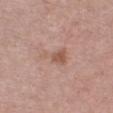- size · ~4 mm (longest diameter)
- TBP lesion metrics · a lesion area of about 6.5 mm², an outline eccentricity of about 0.85 (0 = round, 1 = elongated), and two-axis asymmetry of about 0.5; a border-irregularity index near 5/10 and radial color variation of about 1; a nevus-likeness score of about 5/100 and a detector confidence of about 100 out of 100 that the crop contains a lesion
- body site · the chest
- tile lighting · white-light
- image source · ~15 mm tile from a whole-body skin photo
- patient · female, aged approximately 45